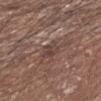Findings:
– workup — catalogued during a skin exam; not biopsied
– image source — total-body-photography crop, ~15 mm field of view
– subject — male, aged 63–67
– size — ≈3 mm
– illumination — white-light
– body site — the left upper arm